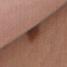Assessment: Captured during whole-body skin photography for melanoma surveillance; the lesion was not biopsied. Acquisition and patient details: From the chest. The recorded lesion diameter is about 6 mm. A female patient, aged approximately 55. This image is a 15 mm lesion crop taken from a total-body photograph.